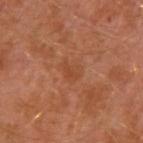Clinical summary:
The lesion is located on the arm. This image is a 15 mm lesion crop taken from a total-body photograph. Longest diameter approximately 2.5 mm. The patient is a male aged around 30.